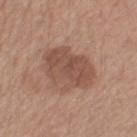Q: Is there a histopathology result?
A: catalogued during a skin exam; not biopsied
Q: What kind of image is this?
A: 15 mm crop, total-body photography
Q: Where on the body is the lesion?
A: the left thigh
Q: Patient demographics?
A: female, about 55 years old